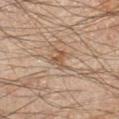This is a cross-polarized tile. A close-up tile cropped from a whole-body skin photograph, about 15 mm across. A male patient, approximately 45 years of age. From the left forearm.